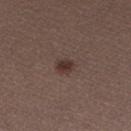Notes:
- notes · catalogued during a skin exam; not biopsied
- patient · female, aged around 30
- lesion diameter · ~2 mm (longest diameter)
- body site · the left lower leg
- acquisition · ~15 mm crop, total-body skin-cancer survey
- TBP lesion metrics · a mean CIELAB color near L≈33 a*≈16 b*≈20 and a lesion–skin lightness drop of about 9; a color-variation rating of about 2.5/10 and radial color variation of about 1; a nevus-likeness score of about 95/100 and a lesion-detection confidence of about 100/100
- tile lighting · white-light